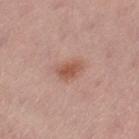Imaged during a routine full-body skin examination; the lesion was not biopsied and no histopathology is available. From the leg. The tile uses white-light illumination. A female subject, aged approximately 55. A lesion tile, about 15 mm wide, cut from a 3D total-body photograph. The recorded lesion diameter is about 3.5 mm.